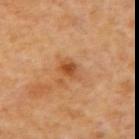Imaged during a routine full-body skin examination; the lesion was not biopsied and no histopathology is available.
From the right upper arm.
A roughly 15 mm field-of-view crop from a total-body skin photograph.
A female patient, aged approximately 55.